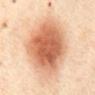Imaged during a routine full-body skin examination; the lesion was not biopsied and no histopathology is available. From the mid back. A female subject, aged 53 to 57. A 15 mm close-up extracted from a 3D total-body photography capture. Automated tile analysis of the lesion measured an area of roughly 45 mm², a shape eccentricity near 0.85, and a shape-asymmetry score of about 0.1 (0 = symmetric). It also reported a lesion color around L≈67 a*≈25 b*≈37 in CIELAB, a lesion–skin lightness drop of about 18, and a normalized border contrast of about 10. And it measured a nevus-likeness score of about 100/100 and lesion-presence confidence of about 100/100.